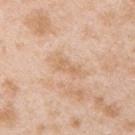Q: Was a biopsy performed?
A: catalogued during a skin exam; not biopsied
Q: What is the anatomic site?
A: the upper back
Q: What did automated image analysis measure?
A: border irregularity of about 3.5 on a 0–10 scale, a within-lesion color-variation index near 1.5/10, and a peripheral color-asymmetry measure near 0.5; a nevus-likeness score of about 0/100 and lesion-presence confidence of about 100/100
Q: Lesion size?
A: about 4 mm
Q: What are the patient's age and sex?
A: male, in their mid- to late 20s
Q: What is the imaging modality?
A: total-body-photography crop, ~15 mm field of view